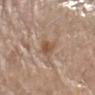follow-up = total-body-photography surveillance lesion; no biopsy | acquisition = ~15 mm crop, total-body skin-cancer survey | lighting = white-light illumination | anatomic site = the left forearm | TBP lesion metrics = a classifier nevus-likeness of about 5/100 and a detector confidence of about 100 out of 100 that the crop contains a lesion | lesion size = ~3.5 mm (longest diameter) | subject = male, roughly 70 years of age.